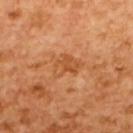Clinical impression: The lesion was tiled from a total-body skin photograph and was not biopsied. Clinical summary: The lesion is located on the upper back. Approximately 3.5 mm at its widest. A female patient, approximately 55 years of age. A lesion tile, about 15 mm wide, cut from a 3D total-body photograph. The tile uses cross-polarized illumination.